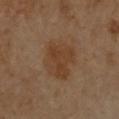Impression: Part of a total-body skin-imaging series; this lesion was reviewed on a skin check and was not flagged for biopsy. Acquisition and patient details: The tile uses cross-polarized illumination. The lesion-visualizer software estimated an area of roughly 16 mm², an eccentricity of roughly 0.7, and two-axis asymmetry of about 0.2. It also reported a mean CIELAB color near L≈40 a*≈18 b*≈31 and about 7 CIELAB-L* units darker than the surrounding skin. And it measured a border-irregularity rating of about 3/10, a within-lesion color-variation index near 3/10, and peripheral color asymmetry of about 1. The analysis additionally found a classifier nevus-likeness of about 90/100 and a lesion-detection confidence of about 100/100. Measured at roughly 5 mm in maximum diameter. From the front of the torso. A roughly 15 mm field-of-view crop from a total-body skin photograph. A female patient roughly 55 years of age.